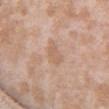Part of a total-body skin-imaging series; this lesion was reviewed on a skin check and was not flagged for biopsy.
The lesion is located on the chest.
A 15 mm close-up tile from a total-body photography series done for melanoma screening.
Imaged with white-light lighting.
A female subject aged 23–27.
About 3 mm across.